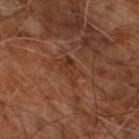follow-up=imaged on a skin check; not biopsied | image=total-body-photography crop, ~15 mm field of view | anatomic site=the right leg | diameter=~3.5 mm (longest diameter) | patient=male, aged around 60.